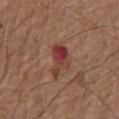Findings:
* biopsy status · imaged on a skin check; not biopsied
* site · the mid back
* acquisition · ~15 mm crop, total-body skin-cancer survey
* TBP lesion metrics · a lesion color around L≈40 a*≈27 b*≈25 in CIELAB, a lesion–skin lightness drop of about 10, and a normalized border contrast of about 8
* patient · male, in their mid-70s